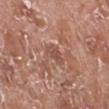Acquisition and patient details: From the right lower leg. A male subject, roughly 75 years of age. The lesion-visualizer software estimated border irregularity of about 3 on a 0–10 scale, a within-lesion color-variation index near 1.5/10, and a peripheral color-asymmetry measure near 0.5. It also reported a nevus-likeness score of about 0/100 and lesion-presence confidence of about 90/100. Cropped from a whole-body photographic skin survey; the tile spans about 15 mm.